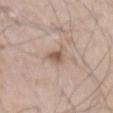notes=imaged on a skin check; not biopsied
diameter=≈3 mm
imaging modality=total-body-photography crop, ~15 mm field of view
location=the abdomen
illumination=white-light
patient=male, aged 58–62